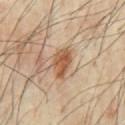Q: Is there a histopathology result?
A: no biopsy performed (imaged during a skin exam)
Q: What is the lesion's diameter?
A: ~4 mm (longest diameter)
Q: What kind of image is this?
A: total-body-photography crop, ~15 mm field of view
Q: Lesion location?
A: the front of the torso
Q: What are the patient's age and sex?
A: male, aged around 65